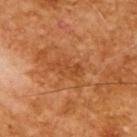{
  "biopsy_status": "not biopsied; imaged during a skin examination",
  "image": {
    "source": "total-body photography crop",
    "field_of_view_mm": 15
  },
  "lighting": "cross-polarized",
  "patient": {
    "sex": "male",
    "age_approx": 65
  }
}